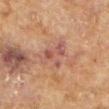The lesion was photographed on a routine skin check and not biopsied; there is no pathology result. Cropped from a whole-body photographic skin survey; the tile spans about 15 mm. The recorded lesion diameter is about 4 mm. Imaged with cross-polarized lighting. The patient is a female roughly 80 years of age. Located on the arm.